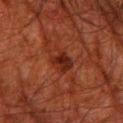  biopsy_status: not biopsied; imaged during a skin examination
  site: leg
  lesion_size:
    long_diameter_mm_approx: 3.5
  image:
    source: total-body photography crop
    field_of_view_mm: 15
  automated_metrics:
    nevus_likeness_0_100: 10
    lesion_detection_confidence_0_100: 100
  patient:
    sex: male
    age_approx: 80